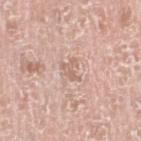Recorded during total-body skin imaging; not selected for excision or biopsy. From the right lower leg. Approximately 2.5 mm at its widest. A male patient, in their mid- to late 70s. A 15 mm close-up tile from a total-body photography series done for melanoma screening. Captured under white-light illumination. The lesion-visualizer software estimated a lesion–skin lightness drop of about 8 and a normalized border contrast of about 5.5. It also reported a classifier nevus-likeness of about 0/100 and a lesion-detection confidence of about 100/100.